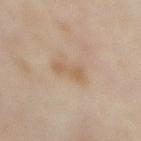follow-up: no biopsy performed (imaged during a skin exam)
subject: female, aged 48–52
anatomic site: the abdomen
imaging modality: ~15 mm tile from a whole-body skin photo
lesion size: ~4 mm (longest diameter)